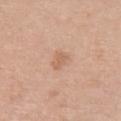Impression: No biopsy was performed on this lesion — it was imaged during a full skin examination and was not determined to be concerning. Clinical summary: Measured at roughly 2.5 mm in maximum diameter. A roughly 15 mm field-of-view crop from a total-body skin photograph. This is a white-light tile. A female subject, in their mid- to late 70s. Automated tile analysis of the lesion measured a mean CIELAB color near L≈61 a*≈21 b*≈32, about 8 CIELAB-L* units darker than the surrounding skin, and a lesion-to-skin contrast of about 5.5 (normalized; higher = more distinct). And it measured an automated nevus-likeness rating near 5 out of 100 and a detector confidence of about 100 out of 100 that the crop contains a lesion. The lesion is on the chest.